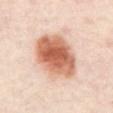Q: Is there a histopathology result?
A: no biopsy performed (imaged during a skin exam)
Q: What did automated image analysis measure?
A: a lesion–skin lightness drop of about 17; a nevus-likeness score of about 100/100 and lesion-presence confidence of about 100/100
Q: Lesion location?
A: the abdomen
Q: Patient demographics?
A: aged around 55
Q: How large is the lesion?
A: about 6.5 mm
Q: How was this image acquired?
A: total-body-photography crop, ~15 mm field of view